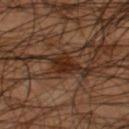The lesion was photographed on a routine skin check and not biopsied; there is no pathology result. A male subject approximately 45 years of age. A 15 mm close-up tile from a total-body photography series done for melanoma screening. This is a cross-polarized tile. Located on the left thigh. About 3 mm across.